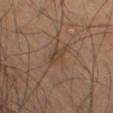Q: Is there a histopathology result?
A: catalogued during a skin exam; not biopsied
Q: What are the patient's age and sex?
A: male, aged 63–67
Q: What is the imaging modality?
A: 15 mm crop, total-body photography
Q: Lesion location?
A: the left thigh